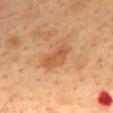Impression: Captured during whole-body skin photography for melanoma surveillance; the lesion was not biopsied. Acquisition and patient details: The lesion's longest dimension is about 4 mm. A roughly 15 mm field-of-view crop from a total-body skin photograph. Imaged with cross-polarized lighting. A male patient in their mid- to late 40s. An algorithmic analysis of the crop reported an area of roughly 7 mm² and an eccentricity of roughly 0.85. The analysis additionally found a border-irregularity index near 3.5/10 and a color-variation rating of about 3/10. The software also gave a nevus-likeness score of about 15/100 and lesion-presence confidence of about 100/100. Located on the mid back.